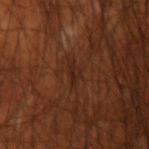workup=no biopsy performed (imaged during a skin exam)
lesion size=≈2.5 mm
image=~15 mm crop, total-body skin-cancer survey
subject=male, aged 68 to 72
illumination=cross-polarized illumination
body site=the right upper arm